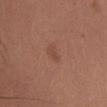Case summary:
– follow-up: no biopsy performed (imaged during a skin exam)
– lighting: white-light
– site: the chest
– acquisition: ~15 mm tile from a whole-body skin photo
– lesion diameter: ≈2.5 mm
– subject: male, aged around 25